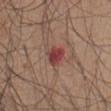<tbp_lesion>
<biopsy_status>not biopsied; imaged during a skin examination</biopsy_status>
</tbp_lesion>